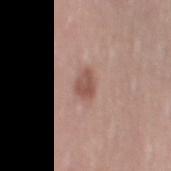Part of a total-body skin-imaging series; this lesion was reviewed on a skin check and was not flagged for biopsy. The lesion's longest dimension is about 3 mm. This image is a 15 mm lesion crop taken from a total-body photograph. A female subject, aged 38–42. The lesion is on the left thigh. Captured under white-light illumination.